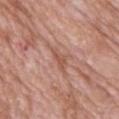Assessment: Part of a total-body skin-imaging series; this lesion was reviewed on a skin check and was not flagged for biopsy. Background: A female subject, aged approximately 70. A roughly 15 mm field-of-view crop from a total-body skin photograph. The lesion is located on the back. The recorded lesion diameter is about 3.5 mm.